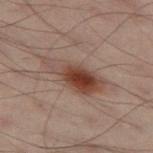Case summary:
– illumination — cross-polarized
– patient — male, roughly 50 years of age
– anatomic site — the left thigh
– automated metrics — an area of roughly 14 mm², an outline eccentricity of about 0.9 (0 = round, 1 = elongated), and a symmetry-axis asymmetry near 0.25; a mean CIELAB color near L≈35 a*≈16 b*≈21, roughly 10 lightness units darker than nearby skin, and a lesion-to-skin contrast of about 9 (normalized; higher = more distinct)
– imaging modality — ~15 mm crop, total-body skin-cancer survey